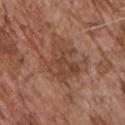follow-up: no biopsy performed (imaged during a skin exam) | automated lesion analysis: a shape-asymmetry score of about 0.35 (0 = symmetric); an average lesion color of about L≈44 a*≈21 b*≈29 (CIELAB), a lesion–skin lightness drop of about 7, and a normalized lesion–skin contrast near 6; a border-irregularity index near 4.5/10 and peripheral color asymmetry of about 1.5; a nevus-likeness score of about 0/100 and lesion-presence confidence of about 95/100 | subject: male, roughly 70 years of age | image source: ~15 mm tile from a whole-body skin photo | site: the chest.